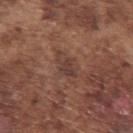Captured during whole-body skin photography for melanoma surveillance; the lesion was not biopsied.
A male subject approximately 75 years of age.
A roughly 15 mm field-of-view crop from a total-body skin photograph.
An algorithmic analysis of the crop reported a border-irregularity rating of about 4/10, a color-variation rating of about 1.5/10, and peripheral color asymmetry of about 0.5. The analysis additionally found a classifier nevus-likeness of about 0/100 and lesion-presence confidence of about 100/100.
Imaged with white-light lighting.
The lesion is on the left upper arm.
Approximately 3.5 mm at its widest.